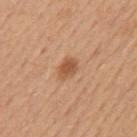<case>
<biopsy_status>not biopsied; imaged during a skin examination</biopsy_status>
<patient>
  <sex>male</sex>
  <age_approx>50</age_approx>
</patient>
<site>mid back</site>
<lesion_size>
  <long_diameter_mm_approx>2.5</long_diameter_mm_approx>
</lesion_size>
<image>
  <source>total-body photography crop</source>
  <field_of_view_mm>15</field_of_view_mm>
</image>
<lighting>white-light</lighting>
<automated_metrics>
  <area_mm2_approx>4.0</area_mm2_approx>
  <eccentricity>0.7</eccentricity>
  <shape_asymmetry>0.3</shape_asymmetry>
  <border_irregularity_0_10>2.5</border_irregularity_0_10>
  <color_variation_0_10>1.5</color_variation_0_10>
  <nevus_likeness_0_100>95</nevus_likeness_0_100>
  <lesion_detection_confidence_0_100>100</lesion_detection_confidence_0_100>
</automated_metrics>
</case>